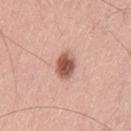Q: Was this lesion biopsied?
A: imaged on a skin check; not biopsied
Q: What kind of image is this?
A: total-body-photography crop, ~15 mm field of view
Q: Lesion location?
A: the left thigh
Q: What are the patient's age and sex?
A: male, aged approximately 50